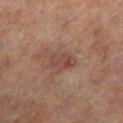Assessment: Imaged during a routine full-body skin examination; the lesion was not biopsied and no histopathology is available.